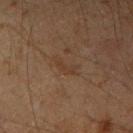Assessment: No biopsy was performed on this lesion — it was imaged during a full skin examination and was not determined to be concerning. Image and clinical context: The tile uses cross-polarized illumination. The patient is a male roughly 50 years of age. Cropped from a whole-body photographic skin survey; the tile spans about 15 mm. The lesion is located on the right upper arm.